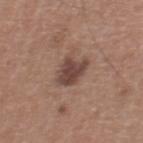biopsy status: total-body-photography surveillance lesion; no biopsy
image source: ~15 mm tile from a whole-body skin photo
body site: the chest
lighting: white-light
lesion diameter: about 3 mm
image-analysis metrics: a lesion color around L≈44 a*≈18 b*≈23 in CIELAB and a normalized border contrast of about 9
subject: male, in their mid-60s